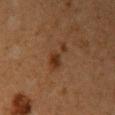Part of a total-body skin-imaging series; this lesion was reviewed on a skin check and was not flagged for biopsy.
This image is a 15 mm lesion crop taken from a total-body photograph.
The lesion is on the arm.
The subject is a female in their 40s.